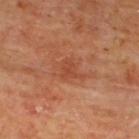The lesion was photographed on a routine skin check and not biopsied; there is no pathology result.
A male subject, in their mid- to late 60s.
The tile uses cross-polarized illumination.
The lesion's longest dimension is about 2.5 mm.
A 15 mm close-up tile from a total-body photography series done for melanoma screening.
From the upper back.